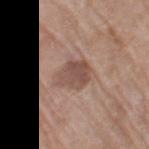biopsy status — imaged on a skin check; not biopsied
TBP lesion metrics — an average lesion color of about L≈50 a*≈18 b*≈25 (CIELAB), a lesion–skin lightness drop of about 10, and a normalized lesion–skin contrast near 7.5; border irregularity of about 2 on a 0–10 scale and a within-lesion color-variation index near 3.5/10
location — the right thigh
tile lighting — white-light
subject — female, roughly 75 years of age
imaging modality — 15 mm crop, total-body photography
size — ~3.5 mm (longest diameter)